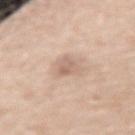Impression: Captured during whole-body skin photography for melanoma surveillance; the lesion was not biopsied. Image and clinical context: A lesion tile, about 15 mm wide, cut from a 3D total-body photograph. Imaged with white-light lighting. Measured at roughly 2.5 mm in maximum diameter. The lesion is located on the right forearm. A female subject aged around 50.